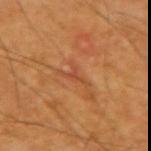– follow-up — no biopsy performed (imaged during a skin exam)
– size — ~3.5 mm (longest diameter)
– site — the arm
– imaging modality — ~15 mm crop, total-body skin-cancer survey
– illumination — cross-polarized
– patient — male, aged approximately 60
– automated lesion analysis — a footprint of about 3.5 mm², a shape eccentricity near 0.85, and a shape-asymmetry score of about 0.7 (0 = symmetric); a classifier nevus-likeness of about 0/100 and a detector confidence of about 85 out of 100 that the crop contains a lesion